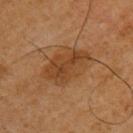Assessment:
Captured during whole-body skin photography for melanoma surveillance; the lesion was not biopsied.
Background:
A male patient, aged approximately 60. The lesion is located on the arm. Automated image analysis of the tile measured an outline eccentricity of about 0.8 (0 = round, 1 = elongated). The analysis additionally found a lesion–skin lightness drop of about 9 and a normalized border contrast of about 7. The software also gave a border-irregularity rating of about 2.5/10 and a peripheral color-asymmetry measure near 1.5. The analysis additionally found a nevus-likeness score of about 10/100. The tile uses cross-polarized illumination. A region of skin cropped from a whole-body photographic capture, roughly 15 mm wide. Longest diameter approximately 6 mm.